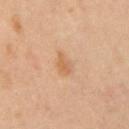* workup · total-body-photography surveillance lesion; no biopsy
* subject · female, about 45 years old
* acquisition · total-body-photography crop, ~15 mm field of view
* body site · the right upper arm